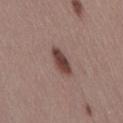notes: total-body-photography surveillance lesion; no biopsy | lighting: white-light illumination | automated metrics: an average lesion color of about L≈42 a*≈19 b*≈21 (CIELAB), roughly 13 lightness units darker than nearby skin, and a lesion-to-skin contrast of about 10.5 (normalized; higher = more distinct); border irregularity of about 1.5 on a 0–10 scale, a within-lesion color-variation index near 3.5/10, and a peripheral color-asymmetry measure near 1; a nevus-likeness score of about 95/100 and lesion-presence confidence of about 100/100 | subject: female, roughly 45 years of age | acquisition: ~15 mm tile from a whole-body skin photo | body site: the right thigh.